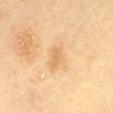image: 15 mm crop, total-body photography
lesion diameter: about 2.5 mm
anatomic site: the abdomen
automated lesion analysis: a shape eccentricity near 0.8 and a shape-asymmetry score of about 0.25 (0 = symmetric); a border-irregularity rating of about 2.5/10, a within-lesion color-variation index near 1/10, and a peripheral color-asymmetry measure near 0.5
tile lighting: cross-polarized
patient: female, aged approximately 55